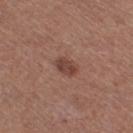Assessment:
No biopsy was performed on this lesion — it was imaged during a full skin examination and was not determined to be concerning.
Clinical summary:
A lesion tile, about 15 mm wide, cut from a 3D total-body photograph. On the leg. The tile uses white-light illumination. Measured at roughly 2.5 mm in maximum diameter. A female patient aged approximately 50.